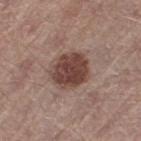{"biopsy_status": "not biopsied; imaged during a skin examination", "lesion_size": {"long_diameter_mm_approx": 4.5}, "image": {"source": "total-body photography crop", "field_of_view_mm": 15}, "patient": {"sex": "male", "age_approx": 55}, "lighting": "white-light", "site": "left thigh"}